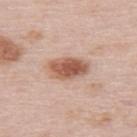Part of a total-body skin-imaging series; this lesion was reviewed on a skin check and was not flagged for biopsy. Approximately 4.5 mm at its widest. An algorithmic analysis of the crop reported a footprint of about 9.5 mm², an eccentricity of roughly 0.85, and a symmetry-axis asymmetry near 0.15. It also reported a detector confidence of about 100 out of 100 that the crop contains a lesion. The subject is a female aged 63–67. A 15 mm close-up extracted from a 3D total-body photography capture. This is a white-light tile. The lesion is on the upper back.